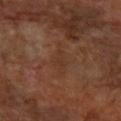| feature | finding |
|---|---|
| biopsy status | catalogued during a skin exam; not biopsied |
| patient | female, aged approximately 65 |
| location | the arm |
| acquisition | total-body-photography crop, ~15 mm field of view |
| lesion size | about 4 mm |
| illumination | cross-polarized |